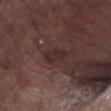<case>
  <biopsy_status>not biopsied; imaged during a skin examination</biopsy_status>
  <image>
    <source>total-body photography crop</source>
    <field_of_view_mm>15</field_of_view_mm>
  </image>
  <site>left lower leg</site>
  <lighting>white-light</lighting>
  <patient>
    <sex>male</sex>
    <age_approx>75</age_approx>
  </patient>
  <lesion_size>
    <long_diameter_mm_approx>3.0</long_diameter_mm_approx>
  </lesion_size>
</case>